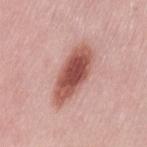  biopsy_status: not biopsied; imaged during a skin examination
  lesion_size:
    long_diameter_mm_approx: 7.0
  patient:
    sex: female
    age_approx: 50
  site: left thigh
  automated_metrics:
    eccentricity: 0.9
    shape_asymmetry: 0.15
    cielab_L: 54
    cielab_a: 26
    cielab_b: 26
    vs_skin_darker_L: 16.0
    vs_skin_contrast_norm: 10.5
    border_irregularity_0_10: 2.5
    color_variation_0_10: 5.5
    peripheral_color_asymmetry: 1.5
  lighting: white-light
  image:
    source: total-body photography crop
    field_of_view_mm: 15